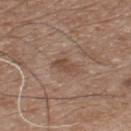notes — imaged on a skin check; not biopsied
lighting — white-light illumination
lesion diameter — ≈3 mm
image source — total-body-photography crop, ~15 mm field of view
subject — male, about 75 years old
body site — the chest